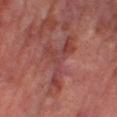workup: catalogued during a skin exam; not biopsied.